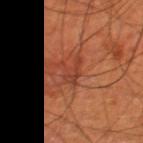biopsy status=total-body-photography surveillance lesion; no biopsy
image=15 mm crop, total-body photography
location=the right thigh
subject=male, roughly 70 years of age